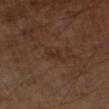  biopsy_status: not biopsied; imaged during a skin examination
  image:
    source: total-body photography crop
    field_of_view_mm: 15
  lesion_size:
    long_diameter_mm_approx: 3.0
  site: left upper arm
  automated_metrics:
    area_mm2_approx: 3.5
    eccentricity: 0.9
    shape_asymmetry: 0.4
    vs_skin_darker_L: 5.0
    vs_skin_contrast_norm: 5.5
    border_irregularity_0_10: 4.5
    color_variation_0_10: 0.5
    peripheral_color_asymmetry: 0.0
    nevus_likeness_0_100: 0
  patient:
    sex: male
    age_approx: 55